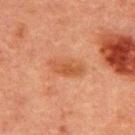biopsy status: catalogued during a skin exam; not biopsied.